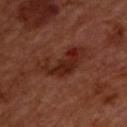Part of a total-body skin-imaging series; this lesion was reviewed on a skin check and was not flagged for biopsy.
An algorithmic analysis of the crop reported an area of roughly 14 mm² and a shape eccentricity near 0.85. The software also gave a lesion color around L≈26 a*≈24 b*≈27 in CIELAB, about 8 CIELAB-L* units darker than the surrounding skin, and a lesion-to-skin contrast of about 8 (normalized; higher = more distinct). It also reported a border-irregularity rating of about 7/10, a within-lesion color-variation index near 5.5/10, and a peripheral color-asymmetry measure near 2. And it measured lesion-presence confidence of about 100/100.
About 6 mm across.
This is a cross-polarized tile.
The lesion is on the upper back.
A male patient, aged around 50.
A roughly 15 mm field-of-view crop from a total-body skin photograph.